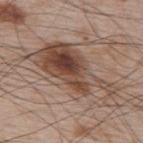<case>
  <biopsy_status>not biopsied; imaged during a skin examination</biopsy_status>
  <site>upper back</site>
  <automated_metrics>
    <cielab_L>47</cielab_L>
    <cielab_a>17</cielab_a>
    <cielab_b>25</cielab_b>
    <vs_skin_contrast_norm>9.5</vs_skin_contrast_norm>
    <border_irregularity_0_10>8.0</border_irregularity_0_10>
    <color_variation_0_10>9.0</color_variation_0_10>
    <peripheral_color_asymmetry>3.0</peripheral_color_asymmetry>
  </automated_metrics>
  <patient>
    <sex>male</sex>
    <age_approx>65</age_approx>
  </patient>
  <lighting>white-light</lighting>
  <image>
    <source>total-body photography crop</source>
    <field_of_view_mm>15</field_of_view_mm>
  </image>
  <lesion_size>
    <long_diameter_mm_approx>11.5</long_diameter_mm_approx>
  </lesion_size>
</case>